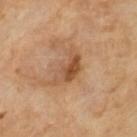  biopsy_status: not biopsied; imaged during a skin examination
  patient:
    sex: male
    age_approx: 65
  lesion_size:
    long_diameter_mm_approx: 5.0
  image:
    source: total-body photography crop
    field_of_view_mm: 15